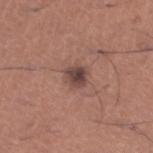workup: total-body-photography surveillance lesion; no biopsy | image-analysis metrics: an area of roughly 4.5 mm², a shape eccentricity near 0.35, and a shape-asymmetry score of about 0.15 (0 = symmetric); a mean CIELAB color near L≈43 a*≈19 b*≈21, about 12 CIELAB-L* units darker than the surrounding skin, and a normalized border contrast of about 10; a border-irregularity rating of about 1.5/10 and a peripheral color-asymmetry measure near 1.5 | diameter: about 2.5 mm | patient: male, aged 43–47 | anatomic site: the left lower leg | imaging modality: ~15 mm crop, total-body skin-cancer survey.